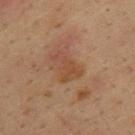Impression:
Part of a total-body skin-imaging series; this lesion was reviewed on a skin check and was not flagged for biopsy.
Background:
Imaged with cross-polarized lighting. The total-body-photography lesion software estimated a border-irregularity rating of about 6.5/10 and radial color variation of about 0.5. The software also gave a nevus-likeness score of about 0/100 and lesion-presence confidence of about 100/100. A roughly 15 mm field-of-view crop from a total-body skin photograph. About 3.5 mm across. A male subject in their mid- to late 30s. The lesion is located on the upper back.